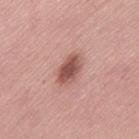Part of a total-body skin-imaging series; this lesion was reviewed on a skin check and was not flagged for biopsy. The lesion's longest dimension is about 4 mm. A close-up tile cropped from a whole-body skin photograph, about 15 mm across. The subject is a female aged approximately 50. The lesion is located on the lower back.